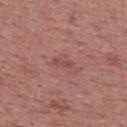Findings:
* biopsy status: catalogued during a skin exam; not biopsied
* image source: ~15 mm tile from a whole-body skin photo
* body site: the upper back
* subject: female, aged around 50